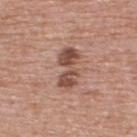Recorded during total-body skin imaging; not selected for excision or biopsy. This image is a 15 mm lesion crop taken from a total-body photograph. A male patient, aged around 70. From the upper back. About 4.5 mm across. The tile uses white-light illumination.